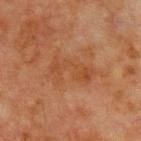Notes:
• biopsy status: catalogued during a skin exam; not biopsied
• imaging modality: 15 mm crop, total-body photography
• lesion size: about 5 mm
• location: the chest
• patient: male, roughly 70 years of age